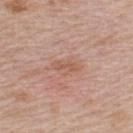Recorded during total-body skin imaging; not selected for excision or biopsy.